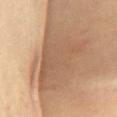No biopsy was performed on this lesion — it was imaged during a full skin examination and was not determined to be concerning. The subject is a female about 50 years old. A 15 mm close-up tile from a total-body photography series done for melanoma screening.